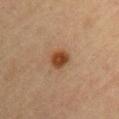Case summary:
- biopsy status — no biopsy performed (imaged during a skin exam)
- automated lesion analysis — a lesion area of about 4.5 mm², an eccentricity of roughly 0.55, and two-axis asymmetry of about 0.25; a border-irregularity index near 2/10, a color-variation rating of about 2.5/10, and radial color variation of about 1; a classifier nevus-likeness of about 100/100 and a detector confidence of about 100 out of 100 that the crop contains a lesion
- site — the right upper arm
- image source — 15 mm crop, total-body photography
- diameter — ~2.5 mm (longest diameter)
- subject — female, aged approximately 65